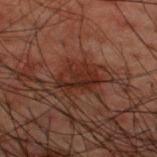Captured during whole-body skin photography for melanoma surveillance; the lesion was not biopsied. The lesion is on the upper back. Measured at roughly 5 mm in maximum diameter. Cropped from a whole-body photographic skin survey; the tile spans about 15 mm. The patient is a male roughly 50 years of age. The tile uses cross-polarized illumination.